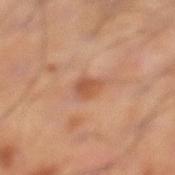Notes:
• notes: no biopsy performed (imaged during a skin exam)